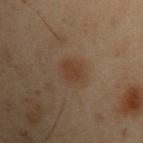Impression: Recorded during total-body skin imaging; not selected for excision or biopsy. Clinical summary: The patient is a male about 55 years old. From the chest. Cropped from a total-body skin-imaging series; the visible field is about 15 mm.